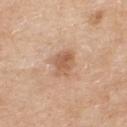Part of a total-body skin-imaging series; this lesion was reviewed on a skin check and was not flagged for biopsy.
A lesion tile, about 15 mm wide, cut from a 3D total-body photograph.
Automated image analysis of the tile measured a footprint of about 6.5 mm², an eccentricity of roughly 0.65, and a shape-asymmetry score of about 0.3 (0 = symmetric). The analysis additionally found a mean CIELAB color near L≈59 a*≈20 b*≈33, about 10 CIELAB-L* units darker than the surrounding skin, and a lesion-to-skin contrast of about 6.5 (normalized; higher = more distinct).
This is a white-light tile.
The lesion is on the upper back.
A male patient, approximately 60 years of age.